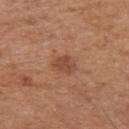{
  "image": {
    "source": "total-body photography crop",
    "field_of_view_mm": 15
  },
  "patient": {
    "sex": "male",
    "age_approx": 65
  },
  "site": "left upper arm"
}